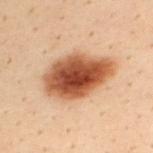The lesion is located on the back. Approximately 7.5 mm at its widest. A male patient, aged approximately 30. A 15 mm crop from a total-body photograph taken for skin-cancer surveillance. The tile uses cross-polarized illumination.